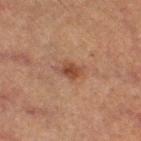Findings:
• biopsy status: imaged on a skin check; not biopsied
• image source: ~15 mm tile from a whole-body skin photo
• subject: female, about 60 years old
• tile lighting: cross-polarized
• lesion diameter: about 3 mm
• location: the right thigh